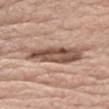Case summary:
– follow-up: total-body-photography surveillance lesion; no biopsy
– subject: female, aged around 70
– automated metrics: an outline eccentricity of about 0.95 (0 = round, 1 = elongated) and a shape-asymmetry score of about 0.25 (0 = symmetric); a lesion color around L≈52 a*≈19 b*≈27 in CIELAB and a lesion-to-skin contrast of about 9.5 (normalized; higher = more distinct); a detector confidence of about 50 out of 100 that the crop contains a lesion
– diameter: ~8 mm (longest diameter)
– imaging modality: ~15 mm tile from a whole-body skin photo
– location: the head or neck
– illumination: white-light